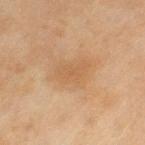Q: Was a biopsy performed?
A: imaged on a skin check; not biopsied
Q: Lesion size?
A: ≈4 mm
Q: Who is the patient?
A: female, about 60 years old
Q: What is the anatomic site?
A: the leg
Q: What kind of image is this?
A: ~15 mm crop, total-body skin-cancer survey